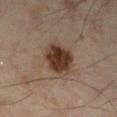notes = imaged on a skin check; not biopsied | automated lesion analysis = a shape eccentricity near 0.5; an average lesion color of about L≈30 a*≈15 b*≈23 (CIELAB) and roughly 12 lightness units darker than nearby skin | patient = male, aged approximately 45 | diameter = ~4 mm (longest diameter) | image = 15 mm crop, total-body photography | body site = the left lower leg.